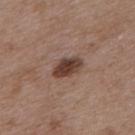Q: Is there a histopathology result?
A: no biopsy performed (imaged during a skin exam)
Q: Where on the body is the lesion?
A: the upper back
Q: What are the patient's age and sex?
A: male, aged 48–52
Q: What kind of image is this?
A: total-body-photography crop, ~15 mm field of view